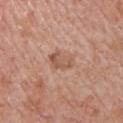  lesion_size:
    long_diameter_mm_approx: 3.0
  site: chest
  image:
    source: total-body photography crop
    field_of_view_mm: 15
  patient:
    sex: male
    age_approx: 75
  lighting: white-light